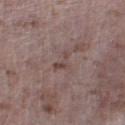Captured during whole-body skin photography for melanoma surveillance; the lesion was not biopsied. Located on the left lower leg. A 15 mm crop from a total-body photograph taken for skin-cancer surveillance. Approximately 3 mm at its widest. The subject is a male approximately 50 years of age. The tile uses white-light illumination.